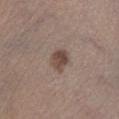The lesion was tiled from a total-body skin photograph and was not biopsied.
A male subject aged around 55.
A 15 mm crop from a total-body photograph taken for skin-cancer surveillance.
Located on the left lower leg.